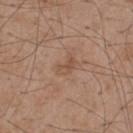follow-up = imaged on a skin check; not biopsied | subject = male, about 50 years old | lighting = white-light | automated metrics = a footprint of about 3.5 mm², an eccentricity of roughly 0.8, and a symmetry-axis asymmetry near 0.4; an automated nevus-likeness rating near 0 out of 100 | image = ~15 mm crop, total-body skin-cancer survey | anatomic site = the back | lesion size = ≈2.5 mm.